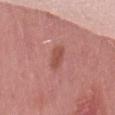Clinical impression: No biopsy was performed on this lesion — it was imaged during a full skin examination and was not determined to be concerning. Image and clinical context: Automated image analysis of the tile measured a footprint of about 3.5 mm². It also reported a lesion color around L≈50 a*≈27 b*≈27 in CIELAB and roughly 9 lightness units darker than nearby skin. And it measured a border-irregularity index near 2.5/10, internal color variation of about 2 on a 0–10 scale, and radial color variation of about 0.5. The software also gave an automated nevus-likeness rating near 30 out of 100 and a detector confidence of about 100 out of 100 that the crop contains a lesion. Captured under white-light illumination. The patient is a male about 40 years old. About 2.5 mm across. Cropped from a total-body skin-imaging series; the visible field is about 15 mm. The lesion is on the lower back.